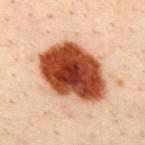Q: Was this lesion biopsied?
A: catalogued during a skin exam; not biopsied
Q: Patient demographics?
A: male, roughly 30 years of age
Q: Lesion size?
A: ~8.5 mm (longest diameter)
Q: Illumination type?
A: cross-polarized illumination
Q: Lesion location?
A: the mid back
Q: What is the imaging modality?
A: total-body-photography crop, ~15 mm field of view